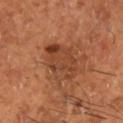* biopsy status · no biopsy performed (imaged during a skin exam)
* lesion size · ~5.5 mm (longest diameter)
* image-analysis metrics · a footprint of about 15 mm², a shape eccentricity near 0.7, and a symmetry-axis asymmetry near 0.2; an average lesion color of about L≈43 a*≈25 b*≈34 (CIELAB), about 8 CIELAB-L* units darker than the surrounding skin, and a lesion-to-skin contrast of about 6.5 (normalized; higher = more distinct); an automated nevus-likeness rating near 55 out of 100
* site · the right lower leg
* subject · male, in their mid- to late 60s
* image · ~15 mm crop, total-body skin-cancer survey